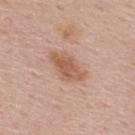{"biopsy_status": "not biopsied; imaged during a skin examination", "image": {"source": "total-body photography crop", "field_of_view_mm": 15}, "site": "upper back", "lesion_size": {"long_diameter_mm_approx": 4.5}, "lighting": "white-light", "patient": {"sex": "male", "age_approx": 55}, "automated_metrics": {"area_mm2_approx": 8.5, "eccentricity": 0.85, "shape_asymmetry": 0.25, "cielab_L": 57, "cielab_a": 22, "cielab_b": 30, "color_variation_0_10": 3.0, "peripheral_color_asymmetry": 1.0, "nevus_likeness_0_100": 40, "lesion_detection_confidence_0_100": 100}}